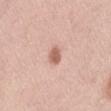Q: How was this image acquired?
A: ~15 mm tile from a whole-body skin photo
Q: Who is the patient?
A: female, in their 50s
Q: How large is the lesion?
A: about 2.5 mm
Q: How was the tile lit?
A: white-light
Q: What is the anatomic site?
A: the right thigh